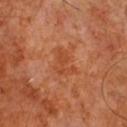biopsy status: no biopsy performed (imaged during a skin exam) | subject: male, aged 58–62 | body site: the front of the torso | imaging modality: 15 mm crop, total-body photography.